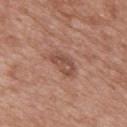  biopsy_status: not biopsied; imaged during a skin examination
  patient:
    sex: male
    age_approx: 50
  lesion_size:
    long_diameter_mm_approx: 3.5
  automated_metrics:
    border_irregularity_0_10: 3.0
    color_variation_0_10: 4.0
    peripheral_color_asymmetry: 1.5
    lesion_detection_confidence_0_100: 100
  image:
    source: total-body photography crop
    field_of_view_mm: 15
  site: mid back